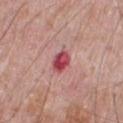Q: Is there a histopathology result?
A: catalogued during a skin exam; not biopsied
Q: Who is the patient?
A: male, aged approximately 70
Q: Where on the body is the lesion?
A: the chest
Q: What is the imaging modality?
A: total-body-photography crop, ~15 mm field of view